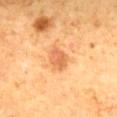Part of a total-body skin-imaging series; this lesion was reviewed on a skin check and was not flagged for biopsy.
Cropped from a total-body skin-imaging series; the visible field is about 15 mm.
The total-body-photography lesion software estimated an area of roughly 6 mm² and two-axis asymmetry of about 0.15. The software also gave border irregularity of about 1.5 on a 0–10 scale and internal color variation of about 3 on a 0–10 scale. And it measured lesion-presence confidence of about 100/100.
Imaged with cross-polarized lighting.
A male subject, aged 58 to 62.
The lesion is located on the mid back.
The lesion's longest dimension is about 3 mm.